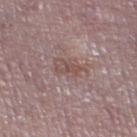No biopsy was performed on this lesion — it was imaged during a full skin examination and was not determined to be concerning.
From the left thigh.
The subject is a male roughly 75 years of age.
A region of skin cropped from a whole-body photographic capture, roughly 15 mm wide.